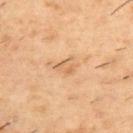The lesion was tiled from a total-body skin photograph and was not biopsied. Longest diameter approximately 2.5 mm. The lesion is on the upper back. A male patient about 55 years old. This image is a 15 mm lesion crop taken from a total-body photograph. Imaged with cross-polarized lighting.